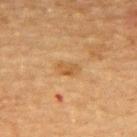Part of a total-body skin-imaging series; this lesion was reviewed on a skin check and was not flagged for biopsy.
A female patient about 70 years old.
On the upper back.
The tile uses cross-polarized illumination.
Cropped from a total-body skin-imaging series; the visible field is about 15 mm.
The lesion's longest dimension is about 3 mm.